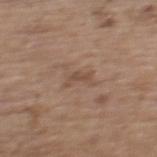The lesion was tiled from a total-body skin photograph and was not biopsied. Measured at roughly 2.5 mm in maximum diameter. Imaged with white-light lighting. The lesion-visualizer software estimated a footprint of about 3 mm² and a symmetry-axis asymmetry near 0.5. It also reported a mean CIELAB color near L≈48 a*≈17 b*≈27 and about 7 CIELAB-L* units darker than the surrounding skin. The software also gave a classifier nevus-likeness of about 0/100 and a detector confidence of about 100 out of 100 that the crop contains a lesion. A lesion tile, about 15 mm wide, cut from a 3D total-body photograph. From the upper back. The patient is a female in their mid- to late 60s.